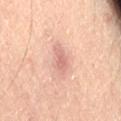Clinical summary: The tile uses cross-polarized illumination. Longest diameter approximately 3.5 mm. From the right thigh. A male subject, approximately 65 years of age. The lesion-visualizer software estimated a lesion area of about 5 mm², an eccentricity of roughly 0.85, and two-axis asymmetry of about 0.3. The software also gave an average lesion color of about L≈65 a*≈24 b*≈26 (CIELAB) and a normalized border contrast of about 6. Cropped from a total-body skin-imaging series; the visible field is about 15 mm.